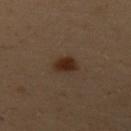Acquisition and patient details:
The tile uses cross-polarized illumination. The lesion's longest dimension is about 2.5 mm. A 15 mm close-up tile from a total-body photography series done for melanoma screening. A female subject aged approximately 50. The lesion is located on the upper back.